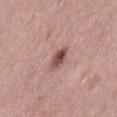Captured during whole-body skin photography for melanoma surveillance; the lesion was not biopsied. Located on the lower back. A female patient, aged 38–42. This image is a 15 mm lesion crop taken from a total-body photograph.